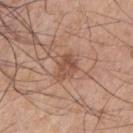| key | value |
|---|---|
| patient | male, about 50 years old |
| size | ~3 mm (longest diameter) |
| acquisition | ~15 mm crop, total-body skin-cancer survey |
| site | the left upper arm |
| lighting | white-light |
| image-analysis metrics | a lesion color around L≈51 a*≈21 b*≈29 in CIELAB and about 9 CIELAB-L* units darker than the surrounding skin |